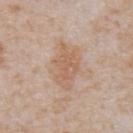The tile uses white-light illumination.
Located on the front of the torso.
A male patient, in their mid- to late 60s.
This image is a 15 mm lesion crop taken from a total-body photograph.
Approximately 5.5 mm at its widest.